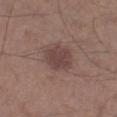Imaged during a routine full-body skin examination; the lesion was not biopsied and no histopathology is available. A male subject, roughly 60 years of age. Approximately 3.5 mm at its widest. This image is a 15 mm lesion crop taken from a total-body photograph. The lesion is on the right lower leg.